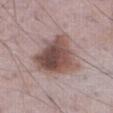The lesion was tiled from a total-body skin photograph and was not biopsied. The tile uses white-light illumination. A 15 mm close-up extracted from a 3D total-body photography capture. Located on the abdomen. Automated tile analysis of the lesion measured a footprint of about 24 mm², an outline eccentricity of about 0.45 (0 = round, 1 = elongated), and a shape-asymmetry score of about 0.35 (0 = symmetric). The software also gave a mean CIELAB color near L≈49 a*≈18 b*≈21 and about 14 CIELAB-L* units darker than the surrounding skin. Approximately 6 mm at its widest. The patient is a male approximately 75 years of age.